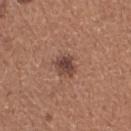biopsy status=total-body-photography surveillance lesion; no biopsy
diameter=≈3 mm
patient=female, aged 33 to 37
body site=the right thigh
image-analysis metrics=an average lesion color of about L≈44 a*≈20 b*≈24 (CIELAB) and a normalized lesion–skin contrast near 9
acquisition=~15 mm tile from a whole-body skin photo